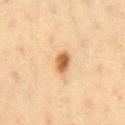Captured during whole-body skin photography for melanoma surveillance; the lesion was not biopsied.
On the right thigh.
A female patient roughly 40 years of age.
The total-body-photography lesion software estimated an area of roughly 4.5 mm² and a shape-asymmetry score of about 0.2 (0 = symmetric). It also reported a classifier nevus-likeness of about 95/100 and a lesion-detection confidence of about 100/100.
This image is a 15 mm lesion crop taken from a total-body photograph.
Imaged with cross-polarized lighting.